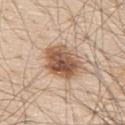No biopsy was performed on this lesion — it was imaged during a full skin examination and was not determined to be concerning. On the upper back. Cropped from a total-body skin-imaging series; the visible field is about 15 mm. A male patient, aged 78–82.